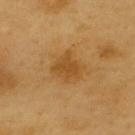workup = total-body-photography surveillance lesion; no biopsy | imaging modality = ~15 mm crop, total-body skin-cancer survey | site = the upper back | patient = male, aged 58 to 62 | tile lighting = cross-polarized | size = ≈3.5 mm.